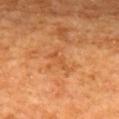- follow-up: total-body-photography surveillance lesion; no biopsy
- anatomic site: the mid back
- patient: male, aged approximately 65
- illumination: cross-polarized
- acquisition: 15 mm crop, total-body photography
- diameter: ~3 mm (longest diameter)